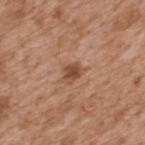image source — 15 mm crop, total-body photography
tile lighting — white-light
body site — the upper back
subject — male, in their mid- to late 60s